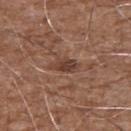Part of a total-body skin-imaging series; this lesion was reviewed on a skin check and was not flagged for biopsy. A male patient, about 75 years old. A region of skin cropped from a whole-body photographic capture, roughly 15 mm wide. The lesion is on the right upper arm.